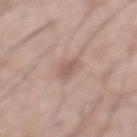The lesion was tiled from a total-body skin photograph and was not biopsied.
A 15 mm crop from a total-body photograph taken for skin-cancer surveillance.
From the front of the torso.
A male patient about 70 years old.
Imaged with white-light lighting.
An algorithmic analysis of the crop reported a footprint of about 4 mm², a shape eccentricity near 0.85, and a shape-asymmetry score of about 0.45 (0 = symmetric). The software also gave a classifier nevus-likeness of about 0/100 and lesion-presence confidence of about 100/100.